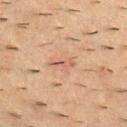Clinical impression:
Part of a total-body skin-imaging series; this lesion was reviewed on a skin check and was not flagged for biopsy.
Context:
A male subject, approximately 55 years of age. The recorded lesion diameter is about 3 mm. A 15 mm close-up extracted from a 3D total-body photography capture. On the upper back.